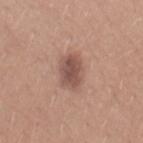Part of a total-body skin-imaging series; this lesion was reviewed on a skin check and was not flagged for biopsy. A female subject aged around 25. Imaged with white-light lighting. A 15 mm close-up tile from a total-body photography series done for melanoma screening. From the front of the torso.